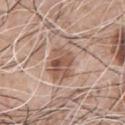No biopsy was performed on this lesion — it was imaged during a full skin examination and was not determined to be concerning. A 15 mm crop from a total-body photograph taken for skin-cancer surveillance. Captured under white-light illumination. Located on the chest. Measured at roughly 5.5 mm in maximum diameter. A male subject, roughly 60 years of age.